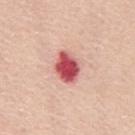Located on the mid back. The patient is a female roughly 70 years of age. A 15 mm close-up tile from a total-body photography series done for melanoma screening.